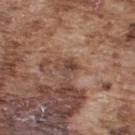The lesion was photographed on a routine skin check and not biopsied; there is no pathology result. Approximately 2.5 mm at its widest. The subject is a male approximately 75 years of age. This is a white-light tile. A 15 mm crop from a total-body photograph taken for skin-cancer surveillance. The lesion is located on the upper back.